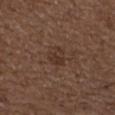No biopsy was performed on this lesion — it was imaged during a full skin examination and was not determined to be concerning. Captured under white-light illumination. Cropped from a total-body skin-imaging series; the visible field is about 15 mm. Automated tile analysis of the lesion measured a footprint of about 4.5 mm² and an eccentricity of roughly 0.7. The software also gave about 6 CIELAB-L* units darker than the surrounding skin and a normalized border contrast of about 6. And it measured internal color variation of about 3 on a 0–10 scale and peripheral color asymmetry of about 1. The recorded lesion diameter is about 2.5 mm. On the left upper arm. A male subject, about 50 years old.